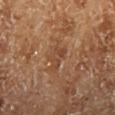Q: Lesion location?
A: the right lower leg
Q: What kind of image is this?
A: ~15 mm crop, total-body skin-cancer survey
Q: What did automated image analysis measure?
A: an area of roughly 4.5 mm², a shape eccentricity near 0.8, and a symmetry-axis asymmetry near 0.4; border irregularity of about 4.5 on a 0–10 scale, a color-variation rating of about 3/10, and radial color variation of about 1; an automated nevus-likeness rating near 0 out of 100 and a lesion-detection confidence of about 100/100
Q: What lighting was used for the tile?
A: cross-polarized illumination
Q: What are the patient's age and sex?
A: male, approximately 70 years of age
Q: How large is the lesion?
A: about 3 mm